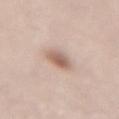Captured during whole-body skin photography for melanoma surveillance; the lesion was not biopsied. This image is a 15 mm lesion crop taken from a total-body photograph. Located on the lower back. The subject is a female in their mid- to late 60s.